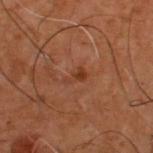follow-up: no biopsy performed (imaged during a skin exam) | subject: male, aged 48 to 52 | image: total-body-photography crop, ~15 mm field of view | automated lesion analysis: a footprint of about 3 mm² and a shape eccentricity near 0.9; an automated nevus-likeness rating near 30 out of 100 | body site: the upper back | tile lighting: cross-polarized illumination.